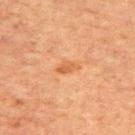Recorded during total-body skin imaging; not selected for excision or biopsy.